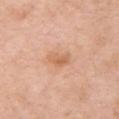Findings:
- workup — total-body-photography surveillance lesion; no biopsy
- anatomic site — the arm
- imaging modality — ~15 mm crop, total-body skin-cancer survey
- illumination — white-light
- image-analysis metrics — an eccentricity of roughly 0.8 and a shape-asymmetry score of about 0.3 (0 = symmetric); lesion-presence confidence of about 100/100
- subject — female, aged around 75